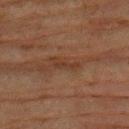notes=total-body-photography surveillance lesion; no biopsy | image=~15 mm tile from a whole-body skin photo | subject=male, aged approximately 80 | location=the arm.